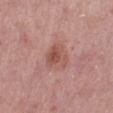Recorded during total-body skin imaging; not selected for excision or biopsy. Cropped from a whole-body photographic skin survey; the tile spans about 15 mm. A female patient aged around 55. The lesion's longest dimension is about 3.5 mm. Imaged with white-light lighting. On the leg. Automated tile analysis of the lesion measured a lesion area of about 7.5 mm².